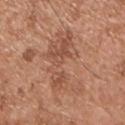<tbp_lesion>
  <biopsy_status>not biopsied; imaged during a skin examination</biopsy_status>
  <image>
    <source>total-body photography crop</source>
    <field_of_view_mm>15</field_of_view_mm>
  </image>
  <lesion_size>
    <long_diameter_mm_approx>8.0</long_diameter_mm_approx>
  </lesion_size>
  <automated_metrics>
    <cielab_L>53</cielab_L>
    <cielab_a>22</cielab_a>
    <cielab_b>31</cielab_b>
    <vs_skin_darker_L>7.0</vs_skin_darker_L>
    <nevus_likeness_0_100>0</nevus_likeness_0_100>
    <lesion_detection_confidence_0_100>100</lesion_detection_confidence_0_100>
  </automated_metrics>
  <site>chest</site>
  <patient>
    <sex>male</sex>
    <age_approx>55</age_approx>
  </patient>
</tbp_lesion>